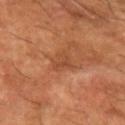* workup · total-body-photography surveillance lesion; no biopsy
* imaging modality · 15 mm crop, total-body photography
* lesion size · ~2.5 mm (longest diameter)
* site · the arm
* TBP lesion metrics · roughly 7 lightness units darker than nearby skin; an automated nevus-likeness rating near 0 out of 100
* patient · male, aged approximately 60
* tile lighting · cross-polarized illumination